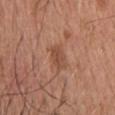{"biopsy_status": "not biopsied; imaged during a skin examination", "lighting": "white-light", "patient": {"sex": "male", "age_approx": 55}, "site": "upper back", "image": {"source": "total-body photography crop", "field_of_view_mm": 15}, "lesion_size": {"long_diameter_mm_approx": 3.0}}